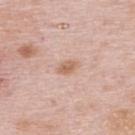Imaged during a routine full-body skin examination; the lesion was not biopsied and no histopathology is available. From the upper back. Approximately 2.5 mm at its widest. Captured under white-light illumination. A region of skin cropped from a whole-body photographic capture, roughly 15 mm wide. A female patient, aged around 65. Automated tile analysis of the lesion measured an outline eccentricity of about 0.8 (0 = round, 1 = elongated) and a symmetry-axis asymmetry near 0.25. The software also gave roughly 10 lightness units darker than nearby skin and a normalized lesion–skin contrast near 7. The software also gave a border-irregularity rating of about 2.5/10 and peripheral color asymmetry of about 1.